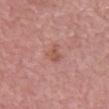The lesion was photographed on a routine skin check and not biopsied; there is no pathology result.